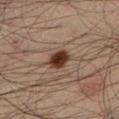  biopsy_status: not biopsied; imaged during a skin examination
  site: left lower leg
  lesion_size:
    long_diameter_mm_approx: 3.0
  image:
    source: total-body photography crop
    field_of_view_mm: 15
  patient:
    sex: male
    age_approx: 35
  lighting: cross-polarized